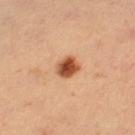{"biopsy_status": "not biopsied; imaged during a skin examination", "site": "leg", "lesion_size": {"long_diameter_mm_approx": 2.5}, "image": {"source": "total-body photography crop", "field_of_view_mm": 15}, "lighting": "cross-polarized", "automated_metrics": {"color_variation_0_10": 5.5, "peripheral_color_asymmetry": 1.5, "nevus_likeness_0_100": 100, "lesion_detection_confidence_0_100": 100}, "patient": {"sex": "female", "age_approx": 35}}